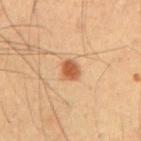  biopsy_status: not biopsied; imaged during a skin examination
  image:
    source: total-body photography crop
    field_of_view_mm: 15
  site: abdomen
  lighting: cross-polarized
  lesion_size:
    long_diameter_mm_approx: 2.5
  automated_metrics:
    area_mm2_approx: 4.5
    eccentricity: 0.55
    shape_asymmetry: 0.25
  patient:
    sex: male
    age_approx: 55